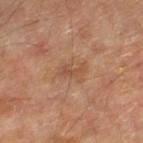The lesion was photographed on a routine skin check and not biopsied; there is no pathology result.
A 15 mm close-up extracted from a 3D total-body photography capture.
Measured at roughly 2.5 mm in maximum diameter.
This is a cross-polarized tile.
The subject is aged 53–57.
The lesion is on the right lower leg.